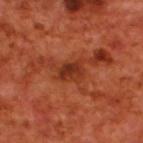notes = catalogued during a skin exam; not biopsied
tile lighting = cross-polarized illumination
site = the back
image source = 15 mm crop, total-body photography
patient = male, aged approximately 70
lesion size = about 3 mm